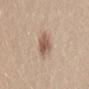Assessment: Recorded during total-body skin imaging; not selected for excision or biopsy. Clinical summary: On the lower back. Automated tile analysis of the lesion measured a footprint of about 7 mm², an eccentricity of roughly 0.3, and two-axis asymmetry of about 0.2. And it measured a lesion color around L≈57 a*≈18 b*≈27 in CIELAB and about 12 CIELAB-L* units darker than the surrounding skin. And it measured an automated nevus-likeness rating near 95 out of 100 and a lesion-detection confidence of about 100/100. The patient is a female roughly 30 years of age. About 3 mm across. A roughly 15 mm field-of-view crop from a total-body skin photograph. Imaged with white-light lighting.